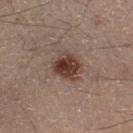Recorded during total-body skin imaging; not selected for excision or biopsy. The subject is a male aged 48–52. The recorded lesion diameter is about 4 mm. Captured under cross-polarized illumination. The lesion is on the left lower leg. A roughly 15 mm field-of-view crop from a total-body skin photograph.